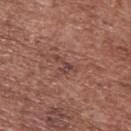{
  "biopsy_status": "not biopsied; imaged during a skin examination",
  "image": {
    "source": "total-body photography crop",
    "field_of_view_mm": 15
  },
  "lesion_size": {
    "long_diameter_mm_approx": 3.0
  },
  "site": "upper back",
  "patient": {
    "sex": "male",
    "age_approx": 75
  }
}